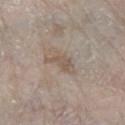Clinical impression:
The lesion was photographed on a routine skin check and not biopsied; there is no pathology result.
Image and clinical context:
Captured under white-light illumination. The recorded lesion diameter is about 4 mm. Automated tile analysis of the lesion measured an area of roughly 5.5 mm² and an outline eccentricity of about 0.85 (0 = round, 1 = elongated). And it measured a border-irregularity index near 5.5/10 and a within-lesion color-variation index near 2.5/10. A roughly 15 mm field-of-view crop from a total-body skin photograph. A male patient aged 58 to 62. The lesion is on the left lower leg.